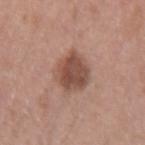Q: Is there a histopathology result?
A: imaged on a skin check; not biopsied
Q: Who is the patient?
A: female, in their 40s
Q: How was this image acquired?
A: ~15 mm tile from a whole-body skin photo
Q: Where on the body is the lesion?
A: the right forearm